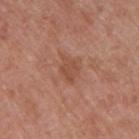- biopsy status — imaged on a skin check; not biopsied